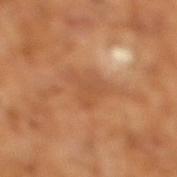Recorded during total-body skin imaging; not selected for excision or biopsy. A male patient, in their mid-60s. This is a cross-polarized tile. A close-up tile cropped from a whole-body skin photograph, about 15 mm across.